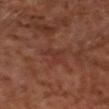workup — no biopsy performed (imaged during a skin exam) | lesion diameter — ~2.5 mm (longest diameter) | anatomic site — the head or neck | subject — male, aged 58–62 | TBP lesion metrics — an area of roughly 3.5 mm², an eccentricity of roughly 0.65, and two-axis asymmetry of about 0.45; a mean CIELAB color near L≈31 a*≈23 b*≈25, a lesion–skin lightness drop of about 4, and a lesion-to-skin contrast of about 4.5 (normalized; higher = more distinct); border irregularity of about 5 on a 0–10 scale, a within-lesion color-variation index near 0.5/10, and a peripheral color-asymmetry measure near 0 | image — ~15 mm crop, total-body skin-cancer survey.